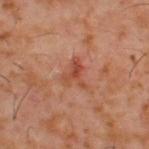Notes:
• image source — ~15 mm crop, total-body skin-cancer survey
• diameter — ~3.5 mm (longest diameter)
• patient — male, in their 60s
• TBP lesion metrics — an area of roughly 5 mm², a shape eccentricity near 0.65, and a shape-asymmetry score of about 0.6 (0 = symmetric); about 8 CIELAB-L* units darker than the surrounding skin; a border-irregularity index near 7/10 and a peripheral color-asymmetry measure near 0.5
• lighting — cross-polarized
• location — the upper back